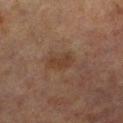{"biopsy_status": "not biopsied; imaged during a skin examination", "lesion_size": {"long_diameter_mm_approx": 3.0}, "patient": {"sex": "male", "age_approx": 70}, "site": "left lower leg", "image": {"source": "total-body photography crop", "field_of_view_mm": 15}, "automated_metrics": {"cielab_L": 31, "cielab_a": 15, "cielab_b": 24, "vs_skin_darker_L": 6.0, "color_variation_0_10": 1.0, "peripheral_color_asymmetry": 0.5}}